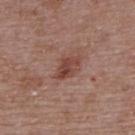follow-up — no biopsy performed (imaged during a skin exam) | imaging modality — total-body-photography crop, ~15 mm field of view | automated lesion analysis — lesion-presence confidence of about 100/100 | patient — female, aged approximately 65 | lighting — white-light illumination | site — the upper back | size — ≈3.5 mm.